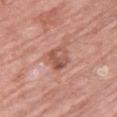Imaged during a routine full-body skin examination; the lesion was not biopsied and no histopathology is available. From the arm. A male patient aged around 80. Automated tile analysis of the lesion measured a footprint of about 6.5 mm², an eccentricity of roughly 0.75, and a shape-asymmetry score of about 0.2 (0 = symmetric). A 15 mm close-up tile from a total-body photography series done for melanoma screening. The tile uses white-light illumination. The recorded lesion diameter is about 3.5 mm.